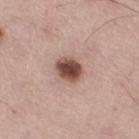| key | value |
|---|---|
| follow-up | no biopsy performed (imaged during a skin exam) |
| lighting | white-light illumination |
| subject | male, aged around 55 |
| image source | total-body-photography crop, ~15 mm field of view |
| site | the right thigh |
| diameter | ≈3 mm |